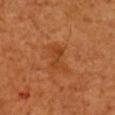workup: imaged on a skin check; not biopsied | subject: male, aged around 50 | illumination: cross-polarized illumination | size: about 3.5 mm | image: ~15 mm tile from a whole-body skin photo | site: the right upper arm.